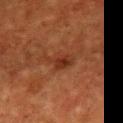<case>
  <biopsy_status>not biopsied; imaged during a skin examination</biopsy_status>
  <image>
    <source>total-body photography crop</source>
    <field_of_view_mm>15</field_of_view_mm>
  </image>
  <patient>
    <sex>female</sex>
    <age_approx>50</age_approx>
  </patient>
  <lighting>cross-polarized</lighting>
  <automated_metrics>
    <nevus_likeness_0_100>5</nevus_likeness_0_100>
  </automated_metrics>
  <site>chest</site>
</case>